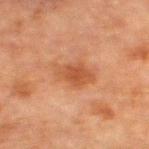Findings:
- workup — imaged on a skin check; not biopsied
- body site — the left thigh
- acquisition — ~15 mm crop, total-body skin-cancer survey
- patient — male, aged around 80
- lesion size — about 4.5 mm
- image-analysis metrics — a mean CIELAB color near L≈42 a*≈22 b*≈31, roughly 8 lightness units darker than nearby skin, and a normalized lesion–skin contrast near 6.5; a border-irregularity index near 3/10, internal color variation of about 3 on a 0–10 scale, and radial color variation of about 1
- illumination — cross-polarized illumination